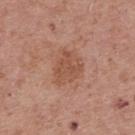Imaged during a routine full-body skin examination; the lesion was not biopsied and no histopathology is available. A 15 mm close-up tile from a total-body photography series done for melanoma screening. The patient is a male in their 70s. The lesion is located on the mid back.